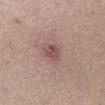notes: no biopsy performed (imaged during a skin exam) | location: the right lower leg | subject: female, about 55 years old | image source: 15 mm crop, total-body photography.